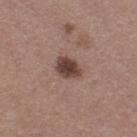Clinical impression: Captured during whole-body skin photography for melanoma surveillance; the lesion was not biopsied. Acquisition and patient details: The tile uses white-light illumination. Located on the right thigh. The patient is a female in their mid- to late 30s. Cropped from a whole-body photographic skin survey; the tile spans about 15 mm.